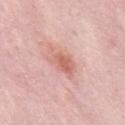  biopsy_status: not biopsied; imaged during a skin examination
  lesion_size:
    long_diameter_mm_approx: 4.5
  site: mid back
  image:
    source: total-body photography crop
    field_of_view_mm: 15
  lighting: white-light
  patient:
    sex: male
    age_approx: 55